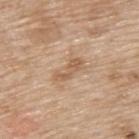The lesion was tiled from a total-body skin photograph and was not biopsied. The lesion-visualizer software estimated border irregularity of about 5 on a 0–10 scale, internal color variation of about 1 on a 0–10 scale, and peripheral color asymmetry of about 0. The software also gave a detector confidence of about 100 out of 100 that the crop contains a lesion. The lesion is located on the upper back. A female subject approximately 75 years of age. This image is a 15 mm lesion crop taken from a total-body photograph. The recorded lesion diameter is about 3.5 mm. The tile uses white-light illumination.